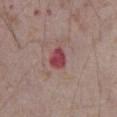notes: catalogued during a skin exam; not biopsied | lesion diameter: ~3 mm (longest diameter) | patient: male, in their mid-70s | location: the abdomen | acquisition: ~15 mm tile from a whole-body skin photo.